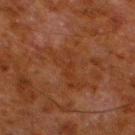workup: catalogued during a skin exam; not biopsied
size: ≈6 mm
subject: male, in their 80s
image: ~15 mm crop, total-body skin-cancer survey
tile lighting: cross-polarized
automated lesion analysis: a footprint of about 11 mm² and an outline eccentricity of about 0.9 (0 = round, 1 = elongated); a lesion color around L≈27 a*≈20 b*≈28 in CIELAB, about 4 CIELAB-L* units darker than the surrounding skin, and a lesion-to-skin contrast of about 4.5 (normalized; higher = more distinct); an automated nevus-likeness rating near 0 out of 100
body site: the left lower leg